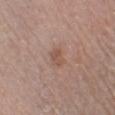This lesion was catalogued during total-body skin photography and was not selected for biopsy. Longest diameter approximately 2.5 mm. The tile uses white-light illumination. A female subject about 70 years old. A 15 mm close-up extracted from a 3D total-body photography capture. From the leg. An algorithmic analysis of the crop reported a footprint of about 3.5 mm², a shape eccentricity near 0.75, and a shape-asymmetry score of about 0.3 (0 = symmetric). It also reported an average lesion color of about L≈52 a*≈20 b*≈27 (CIELAB) and roughly 7 lightness units darker than nearby skin. It also reported a classifier nevus-likeness of about 0/100 and a lesion-detection confidence of about 100/100.